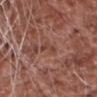The lesion was photographed on a routine skin check and not biopsied; there is no pathology result.
Located on the head or neck.
A male patient, approximately 75 years of age.
Longest diameter approximately 3 mm.
A 15 mm crop from a total-body photograph taken for skin-cancer surveillance.
Automated image analysis of the tile measured an area of roughly 2.5 mm² and a shape eccentricity near 0.95. The software also gave a lesion color around L≈42 a*≈23 b*≈26 in CIELAB and roughly 7 lightness units darker than nearby skin. And it measured a border-irregularity index near 6/10, a color-variation rating of about 0/10, and a peripheral color-asymmetry measure near 0.